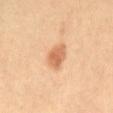| field | value |
|---|---|
| biopsy status | imaged on a skin check; not biopsied |
| image source | ~15 mm tile from a whole-body skin photo |
| body site | the abdomen |
| illumination | cross-polarized |
| patient | male, aged 38–42 |